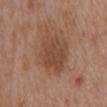biopsy status: catalogued during a skin exam; not biopsied
subject: male, in their mid- to late 70s
TBP lesion metrics: an area of roughly 16 mm², an eccentricity of roughly 0.7, and a symmetry-axis asymmetry near 0.15; an average lesion color of about L≈45 a*≈20 b*≈29 (CIELAB), about 8 CIELAB-L* units darker than the surrounding skin, and a normalized border contrast of about 6.5
tile lighting: white-light illumination
lesion diameter: ~5.5 mm (longest diameter)
image source: total-body-photography crop, ~15 mm field of view
anatomic site: the mid back